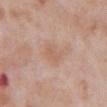acquisition: total-body-photography crop, ~15 mm field of view | subject: male, in their mid- to late 50s | body site: the abdomen.